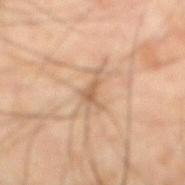Imaged during a routine full-body skin examination; the lesion was not biopsied and no histopathology is available. Longest diameter approximately 3.5 mm. An algorithmic analysis of the crop reported an area of roughly 3.5 mm² and an eccentricity of roughly 0.85. The analysis additionally found about 9 CIELAB-L* units darker than the surrounding skin and a normalized border contrast of about 6. And it measured an automated nevus-likeness rating near 0 out of 100. A 15 mm close-up tile from a total-body photography series done for melanoma screening. A male patient about 65 years old. On the abdomen.